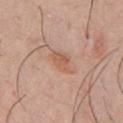Notes:
• workup — total-body-photography surveillance lesion; no biopsy
• tile lighting — white-light illumination
• subject — male, about 30 years old
• lesion size — ≈3.5 mm
• automated lesion analysis — a lesion area of about 4.5 mm², a shape eccentricity near 0.85, and a shape-asymmetry score of about 0.25 (0 = symmetric); a border-irregularity rating of about 2.5/10 and internal color variation of about 2 on a 0–10 scale
• site — the front of the torso
• image — 15 mm crop, total-body photography